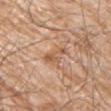  biopsy_status: not biopsied; imaged during a skin examination
  image:
    source: total-body photography crop
    field_of_view_mm: 15
  patient:
    sex: male
    age_approx: 80
  automated_metrics:
    area_mm2_approx: 4.5
    eccentricity: 0.8
    shape_asymmetry: 0.4
    border_irregularity_0_10: 5.5
    color_variation_0_10: 2.5
    peripheral_color_asymmetry: 1.0
    nevus_likeness_0_100: 0
  site: left upper arm